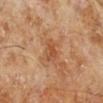Approximately 3.5 mm at its widest. The lesion is located on the left lower leg. Captured under cross-polarized illumination. A male patient aged 68 to 72. Cropped from a whole-body photographic skin survey; the tile spans about 15 mm.